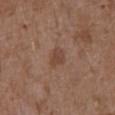The subject is a male aged 73–77.
The lesion is on the abdomen.
Captured under white-light illumination.
A 15 mm close-up tile from a total-body photography series done for melanoma screening.